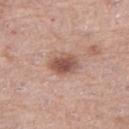Clinical impression:
This lesion was catalogued during total-body skin photography and was not selected for biopsy.
Background:
Automated tile analysis of the lesion measured roughly 13 lightness units darker than nearby skin and a normalized lesion–skin contrast near 9. The software also gave border irregularity of about 1.5 on a 0–10 scale, internal color variation of about 3.5 on a 0–10 scale, and peripheral color asymmetry of about 1. This image is a 15 mm lesion crop taken from a total-body photograph. From the right thigh. The patient is a female approximately 65 years of age. The recorded lesion diameter is about 3.5 mm. This is a white-light tile.